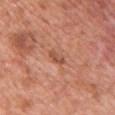workup: imaged on a skin check; not biopsied | subject: female, aged around 50 | automated metrics: an outline eccentricity of about 0.9 (0 = round, 1 = elongated) and a symmetry-axis asymmetry near 0.35; a mean CIELAB color near L≈52 a*≈26 b*≈32 and a lesion-to-skin contrast of about 6.5 (normalized; higher = more distinct); a nevus-likeness score of about 0/100 and lesion-presence confidence of about 100/100 | acquisition: ~15 mm tile from a whole-body skin photo | tile lighting: white-light illumination | body site: the chest.